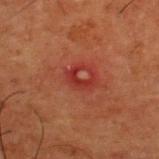Imaged during a routine full-body skin examination; the lesion was not biopsied and no histopathology is available. The lesion-visualizer software estimated a footprint of about 3 mm² and two-axis asymmetry of about 0.6. A 15 mm close-up extracted from a 3D total-body photography capture. A male subject, about 50 years old. Captured under cross-polarized illumination. The lesion is on the upper back. The recorded lesion diameter is about 2.5 mm.